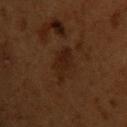Assessment: Part of a total-body skin-imaging series; this lesion was reviewed on a skin check and was not flagged for biopsy. Context: About 4 mm across. The lesion is on the chest. This image is a 15 mm lesion crop taken from a total-body photograph. A male subject, in their 50s. The tile uses cross-polarized illumination.